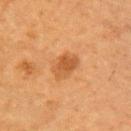Part of a total-body skin-imaging series; this lesion was reviewed on a skin check and was not flagged for biopsy.
The lesion is on the right upper arm.
A lesion tile, about 15 mm wide, cut from a 3D total-body photograph.
A male subject, in their mid-80s or older.
Captured under cross-polarized illumination.
The total-body-photography lesion software estimated an average lesion color of about L≈48 a*≈23 b*≈39 (CIELAB), a lesion–skin lightness drop of about 9, and a normalized border contrast of about 7. And it measured a border-irregularity index near 2/10 and a color-variation rating of about 3/10. The analysis additionally found a lesion-detection confidence of about 100/100.